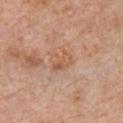Captured during whole-body skin photography for melanoma surveillance; the lesion was not biopsied.
Measured at roughly 3 mm in maximum diameter.
On the chest.
A 15 mm close-up extracted from a 3D total-body photography capture.
Imaged with white-light lighting.
The patient is a male aged around 60.